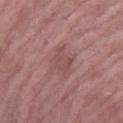follow-up: catalogued during a skin exam; not biopsied
patient: female, aged approximately 60
image-analysis metrics: an average lesion color of about L≈50 a*≈22 b*≈22 (CIELAB), about 6 CIELAB-L* units darker than the surrounding skin, and a lesion-to-skin contrast of about 5 (normalized; higher = more distinct); a border-irregularity rating of about 4/10 and radial color variation of about 0.5
tile lighting: white-light illumination
anatomic site: the left forearm
diameter: about 4 mm
acquisition: ~15 mm tile from a whole-body skin photo